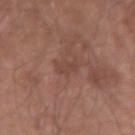Q: Was this lesion biopsied?
A: catalogued during a skin exam; not biopsied
Q: Who is the patient?
A: male, aged approximately 70
Q: What is the anatomic site?
A: the left forearm
Q: Illumination type?
A: white-light
Q: How was this image acquired?
A: total-body-photography crop, ~15 mm field of view
Q: How large is the lesion?
A: ~3 mm (longest diameter)